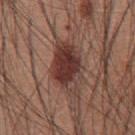Case summary:
- notes — catalogued during a skin exam; not biopsied